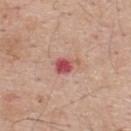Approximately 3 mm at its widest.
The lesion is located on the upper back.
Captured under white-light illumination.
A close-up tile cropped from a whole-body skin photograph, about 15 mm across.
A male subject, roughly 60 years of age.
The lesion-visualizer software estimated a shape eccentricity near 0.8 and a symmetry-axis asymmetry near 0.35. It also reported about 13 CIELAB-L* units darker than the surrounding skin. The software also gave a border-irregularity rating of about 3.5/10, a within-lesion color-variation index near 2.5/10, and radial color variation of about 1.